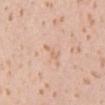This lesion was catalogued during total-body skin photography and was not selected for biopsy.
A lesion tile, about 15 mm wide, cut from a 3D total-body photograph.
The lesion is on the right upper arm.
The subject is a female aged 18 to 22.
An algorithmic analysis of the crop reported an area of roughly 2 mm², an eccentricity of roughly 0.95, and a shape-asymmetry score of about 0.55 (0 = symmetric). The analysis additionally found roughly 6 lightness units darker than nearby skin and a lesion-to-skin contrast of about 5 (normalized; higher = more distinct). And it measured border irregularity of about 5.5 on a 0–10 scale, a color-variation rating of about 0/10, and peripheral color asymmetry of about 0. The analysis additionally found a classifier nevus-likeness of about 0/100 and a lesion-detection confidence of about 100/100.
Longest diameter approximately 2.5 mm.
The tile uses white-light illumination.